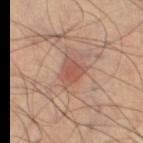notes: no biopsy performed (imaged during a skin exam)
patient: male, in their mid-40s
automated lesion analysis: an average lesion color of about L≈51 a*≈23 b*≈27 (CIELAB), a lesion–skin lightness drop of about 8, and a lesion-to-skin contrast of about 6 (normalized; higher = more distinct)
illumination: cross-polarized
lesion size: ~3 mm (longest diameter)
anatomic site: the left lower leg
acquisition: ~15 mm crop, total-body skin-cancer survey